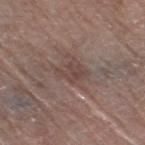Assessment: Part of a total-body skin-imaging series; this lesion was reviewed on a skin check and was not flagged for biopsy. Context: Measured at roughly 3 mm in maximum diameter. The patient is a female aged around 80. The tile uses white-light illumination. A 15 mm close-up tile from a total-body photography series done for melanoma screening. The lesion is located on the leg.